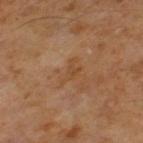| field | value |
|---|---|
| notes | no biopsy performed (imaged during a skin exam) |
| anatomic site | the leg |
| diameter | ~3 mm (longest diameter) |
| automated metrics | a footprint of about 4 mm² and a symmetry-axis asymmetry near 0.55; a border-irregularity rating of about 5.5/10, a within-lesion color-variation index near 1/10, and peripheral color asymmetry of about 0.5 |
| tile lighting | cross-polarized |
| imaging modality | ~15 mm tile from a whole-body skin photo |
| patient | male, about 60 years old |